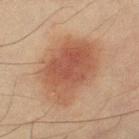- follow-up — catalogued during a skin exam; not biopsied
- image — 15 mm crop, total-body photography
- location — the left thigh
- subject — female, aged 53–57
- automated metrics — about 9 CIELAB-L* units darker than the surrounding skin; a border-irregularity rating of about 2.5/10, internal color variation of about 4.5 on a 0–10 scale, and peripheral color asymmetry of about 1.5
- illumination — cross-polarized
- diameter — about 8 mm